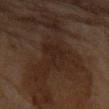Notes:
* follow-up · no biopsy performed (imaged during a skin exam)
* subject · female, about 80 years old
* automated lesion analysis · an average lesion color of about L≈18 a*≈13 b*≈19 (CIELAB), roughly 4 lightness units darker than nearby skin, and a normalized lesion–skin contrast near 5.5; a border-irregularity index near 4.5/10, a color-variation rating of about 2/10, and radial color variation of about 0.5
* imaging modality · ~15 mm tile from a whole-body skin photo
* body site · the left forearm